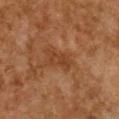Recorded during total-body skin imaging; not selected for excision or biopsy. Automated tile analysis of the lesion measured a mean CIELAB color near L≈36 a*≈21 b*≈31, roughly 6 lightness units darker than nearby skin, and a lesion-to-skin contrast of about 6 (normalized; higher = more distinct). The analysis additionally found internal color variation of about 2 on a 0–10 scale and radial color variation of about 0.5. This is a cross-polarized tile. A region of skin cropped from a whole-body photographic capture, roughly 15 mm wide. About 4 mm across. A female patient, aged around 60. Located on the chest.